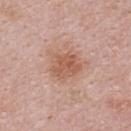notes: catalogued during a skin exam; not biopsied | location: the back | image: 15 mm crop, total-body photography | illumination: white-light | image-analysis metrics: a shape-asymmetry score of about 0.2 (0 = symmetric); a classifier nevus-likeness of about 25/100 and a lesion-detection confidence of about 100/100 | subject: female, approximately 30 years of age | lesion diameter: about 3.5 mm.